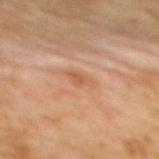follow-up: total-body-photography surveillance lesion; no biopsy | site: the back | subject: female, aged approximately 70 | image: 15 mm crop, total-body photography.